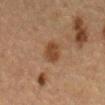illumination — cross-polarized
image — 15 mm crop, total-body photography
automated lesion analysis — an area of roughly 6 mm², a shape eccentricity near 0.8, and a symmetry-axis asymmetry near 0.25; a nevus-likeness score of about 90/100 and a lesion-detection confidence of about 100/100
anatomic site — the abdomen
subject — male, about 65 years old